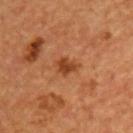The lesion was tiled from a total-body skin photograph and was not biopsied. The lesion's longest dimension is about 2.5 mm. From the front of the torso. A male patient, aged around 55. Automated image analysis of the tile measured a border-irregularity index near 3/10, a within-lesion color-variation index near 1.5/10, and radial color variation of about 0.5. The software also gave a nevus-likeness score of about 15/100 and lesion-presence confidence of about 100/100. A 15 mm close-up tile from a total-body photography series done for melanoma screening.